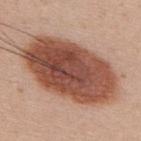* workup: total-body-photography surveillance lesion; no biopsy
* subject: male, aged 33–37
* site: the upper back
* image-analysis metrics: a footprint of about 55 mm², an eccentricity of roughly 0.85, and a shape-asymmetry score of about 0.1 (0 = symmetric); border irregularity of about 1.5 on a 0–10 scale, a color-variation rating of about 7/10, and peripheral color asymmetry of about 2.5; an automated nevus-likeness rating near 100 out of 100 and a lesion-detection confidence of about 100/100
* lesion diameter: ≈11.5 mm
* tile lighting: white-light
* image: ~15 mm crop, total-body skin-cancer survey